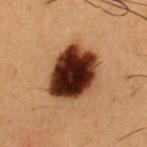biopsy status: total-body-photography surveillance lesion; no biopsy
image: ~15 mm tile from a whole-body skin photo
patient: male, aged 48 to 52
body site: the chest